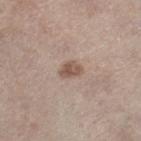• notes · no biopsy performed (imaged during a skin exam)
• imaging modality · total-body-photography crop, ~15 mm field of view
• patient · male, aged around 70
• location · the right lower leg
• lesion diameter · ~2.5 mm (longest diameter)